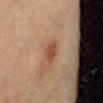A roughly 15 mm field-of-view crop from a total-body skin photograph. The lesion is on the abdomen. The lesion-visualizer software estimated a footprint of about 4 mm² and a symmetry-axis asymmetry near 0.2. And it measured border irregularity of about 1.5 on a 0–10 scale and a color-variation rating of about 1.5/10. And it measured a classifier nevus-likeness of about 85/100 and a lesion-detection confidence of about 100/100. A female subject approximately 40 years of age. Approximately 2.5 mm at its widest. Captured under cross-polarized illumination.